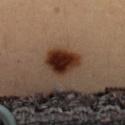Part of a total-body skin-imaging series; this lesion was reviewed on a skin check and was not flagged for biopsy. The lesion is located on the upper back. The recorded lesion diameter is about 4 mm. A close-up tile cropped from a whole-body skin photograph, about 15 mm across. Imaged with cross-polarized lighting. A female patient, aged approximately 35.